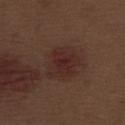Case summary:
* workup — no biopsy performed (imaged during a skin exam)
* patient — male, aged around 70
* lesion size — ~2.5 mm (longest diameter)
* lighting — white-light illumination
* image — 15 mm crop, total-body photography
* location — the leg
* TBP lesion metrics — a border-irregularity rating of about 2.5/10 and peripheral color asymmetry of about 1; an automated nevus-likeness rating near 0 out of 100 and a lesion-detection confidence of about 100/100